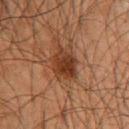workup = catalogued during a skin exam; not biopsied
lesion diameter = about 3.5 mm
image = ~15 mm tile from a whole-body skin photo
patient = male, aged 63–67
automated metrics = a footprint of about 8 mm² and a symmetry-axis asymmetry near 0.2; a lesion color around L≈30 a*≈20 b*≈28 in CIELAB; a classifier nevus-likeness of about 75/100 and a lesion-detection confidence of about 100/100
location = the front of the torso